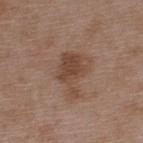Recorded during total-body skin imaging; not selected for excision or biopsy.
Automated image analysis of the tile measured a border-irregularity index near 5.5/10, a within-lesion color-variation index near 2.5/10, and radial color variation of about 1.
On the back.
A 15 mm close-up extracted from a 3D total-body photography capture.
The subject is a male approximately 50 years of age.